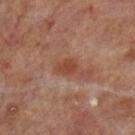{
  "biopsy_status": "not biopsied; imaged during a skin examination",
  "patient": {
    "sex": "male",
    "age_approx": 70
  },
  "site": "left lower leg",
  "image": {
    "source": "total-body photography crop",
    "field_of_view_mm": 15
  }
}